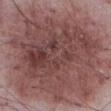• biopsy status · no biopsy performed (imaged during a skin exam)
• tile lighting · white-light illumination
• patient · male, aged around 55
• acquisition · total-body-photography crop, ~15 mm field of view
• diameter · ~15.5 mm (longest diameter)
• body site · the abdomen
• automated metrics · a lesion color around L≈46 a*≈21 b*≈20 in CIELAB and a normalized border contrast of about 9.5; a color-variation rating of about 6.5/10 and radial color variation of about 2.5; an automated nevus-likeness rating near 20 out of 100 and a detector confidence of about 95 out of 100 that the crop contains a lesion